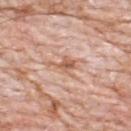Impression:
No biopsy was performed on this lesion — it was imaged during a full skin examination and was not determined to be concerning.
Background:
A male patient, aged around 80. Located on the upper back. A 15 mm crop from a total-body photograph taken for skin-cancer surveillance. The total-body-photography lesion software estimated an area of roughly 5 mm² and a shape eccentricity near 0.85. It also reported a mean CIELAB color near L≈61 a*≈22 b*≈31 and roughly 9 lightness units darker than nearby skin. And it measured a border-irregularity rating of about 7/10, internal color variation of about 3.5 on a 0–10 scale, and peripheral color asymmetry of about 1. The lesion's longest dimension is about 4 mm. The tile uses white-light illumination.